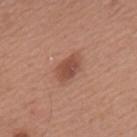This lesion was catalogued during total-body skin photography and was not selected for biopsy. A male subject, roughly 65 years of age. Approximately 3.5 mm at its widest. On the mid back. The lesion-visualizer software estimated a footprint of about 6.5 mm², an eccentricity of roughly 0.8, and two-axis asymmetry of about 0.25. The software also gave an average lesion color of about L≈49 a*≈23 b*≈29 (CIELAB). A region of skin cropped from a whole-body photographic capture, roughly 15 mm wide.